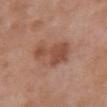No biopsy was performed on this lesion — it was imaged during a full skin examination and was not determined to be concerning. Located on the chest. Automated image analysis of the tile measured a mean CIELAB color near L≈49 a*≈22 b*≈30, a lesion–skin lightness drop of about 10, and a normalized border contrast of about 7.5. The software also gave a border-irregularity rating of about 6/10 and a color-variation rating of about 2/10. This is a white-light tile. A 15 mm crop from a total-body photograph taken for skin-cancer surveillance. A female subject approximately 70 years of age.